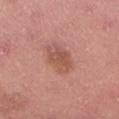A 15 mm close-up tile from a total-body photography series done for melanoma screening.
On the back.
The tile uses white-light illumination.
A male patient roughly 40 years of age.